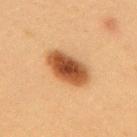Case summary:
• notes: no biopsy performed (imaged during a skin exam)
• body site: the upper back
• image source: ~15 mm crop, total-body skin-cancer survey
• patient: female, aged 38–42